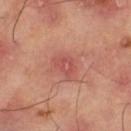biopsy_status: not biopsied; imaged during a skin examination
site: right thigh
image:
  source: total-body photography crop
  field_of_view_mm: 15
lesion_size:
  long_diameter_mm_approx: 3.0
lighting: cross-polarized